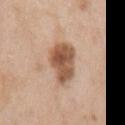notes = no biopsy performed (imaged during a skin exam)
subject = female, aged 38–42
body site = the arm
tile lighting = white-light illumination
diameter = ≈5 mm
imaging modality = total-body-photography crop, ~15 mm field of view